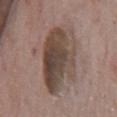Imaged during a routine full-body skin examination; the lesion was not biopsied and no histopathology is available.
Captured under white-light illumination.
The total-body-photography lesion software estimated a mean CIELAB color near L≈44 a*≈13 b*≈22 and roughly 12 lightness units darker than nearby skin. The analysis additionally found a border-irregularity rating of about 3/10, a color-variation rating of about 8.5/10, and radial color variation of about 3.
The subject is a male about 75 years old.
Cropped from a total-body skin-imaging series; the visible field is about 15 mm.
On the mid back.
About 8 mm across.